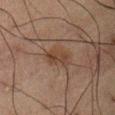workup: total-body-photography surveillance lesion; no biopsy | image: 15 mm crop, total-body photography | location: the front of the torso | lighting: cross-polarized illumination | automated lesion analysis: a border-irregularity index near 3.5/10 and a peripheral color-asymmetry measure near 1.5 | patient: male, about 60 years old.